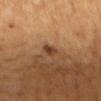Recorded during total-body skin imaging; not selected for excision or biopsy. The lesion's longest dimension is about 2 mm. This is a cross-polarized tile. Located on the back. A female subject. A roughly 15 mm field-of-view crop from a total-body skin photograph.